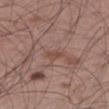The lesion was tiled from a total-body skin photograph and was not biopsied. This image is a 15 mm lesion crop taken from a total-body photograph. A male patient roughly 55 years of age. The lesion-visualizer software estimated a lesion–skin lightness drop of about 6 and a lesion-to-skin contrast of about 5 (normalized; higher = more distinct). The analysis additionally found a border-irregularity index near 2.5/10, a within-lesion color-variation index near 2/10, and radial color variation of about 0.5. The analysis additionally found a classifier nevus-likeness of about 0/100. The lesion is located on the leg. This is a white-light tile.